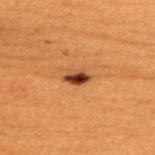<lesion>
  <biopsy_status>not biopsied; imaged during a skin examination</biopsy_status>
  <patient>
    <sex>male</sex>
    <age_approx>50</age_approx>
  </patient>
  <image>
    <source>total-body photography crop</source>
    <field_of_view_mm>15</field_of_view_mm>
  </image>
  <site>upper back</site>
  <automated_metrics>
    <area_mm2_approx>4.0</area_mm2_approx>
    <shape_asymmetry>0.2</shape_asymmetry>
    <vs_skin_darker_L>14.0</vs_skin_darker_L>
  </automated_metrics>
  <lesion_size>
    <long_diameter_mm_approx>3.0</long_diameter_mm_approx>
  </lesion_size>
  <lighting>cross-polarized</lighting>
</lesion>